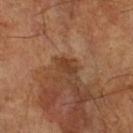Imaged during a routine full-body skin examination; the lesion was not biopsied and no histopathology is available.
The recorded lesion diameter is about 3 mm.
A region of skin cropped from a whole-body photographic capture, roughly 15 mm wide.
The subject is a male roughly 65 years of age.
The total-body-photography lesion software estimated a mean CIELAB color near L≈38 a*≈20 b*≈31, a lesion–skin lightness drop of about 8, and a normalized border contrast of about 6.5. The analysis additionally found border irregularity of about 2 on a 0–10 scale.